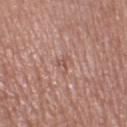follow-up = total-body-photography surveillance lesion; no biopsy
imaging modality = 15 mm crop, total-body photography
tile lighting = white-light illumination
size = ~3 mm (longest diameter)
site = the right thigh
patient = female, aged around 40